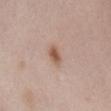This lesion was catalogued during total-body skin photography and was not selected for biopsy. Cropped from a total-body skin-imaging series; the visible field is about 15 mm. The subject is a female aged 48–52. From the front of the torso. Imaged with white-light lighting. The lesion's longest dimension is about 2.5 mm.